Located on the upper back · the subject is a male aged approximately 80 · cropped from a total-body skin-imaging series; the visible field is about 15 mm.
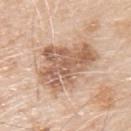The biopsy diagnosis was a melanoma in situ, lentigo maligna type — a malignant skin lesion.